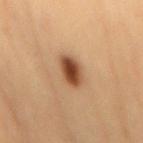| field | value |
|---|---|
| notes | no biopsy performed (imaged during a skin exam) |
| image | ~15 mm crop, total-body skin-cancer survey |
| size | ≈3.5 mm |
| image-analysis metrics | an average lesion color of about L≈47 a*≈21 b*≈34 (CIELAB), a lesion–skin lightness drop of about 16, and a lesion-to-skin contrast of about 11.5 (normalized; higher = more distinct); a border-irregularity rating of about 2/10, a color-variation rating of about 5/10, and peripheral color asymmetry of about 1.5; a nevus-likeness score of about 100/100 and a detector confidence of about 100 out of 100 that the crop contains a lesion |
| subject | female, roughly 65 years of age |
| body site | the mid back |